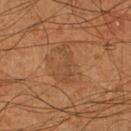subject = male, in their mid-50s; site = the right lower leg; image = total-body-photography crop, ~15 mm field of view; illumination = cross-polarized illumination; diameter = about 4.5 mm.